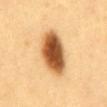Impression:
The lesion was photographed on a routine skin check and not biopsied; there is no pathology result.
Clinical summary:
The lesion is on the front of the torso. Captured under cross-polarized illumination. A region of skin cropped from a whole-body photographic capture, roughly 15 mm wide. Approximately 6 mm at its widest. A male subject, in their 60s. The total-body-photography lesion software estimated a lesion color around L≈55 a*≈23 b*≈42 in CIELAB, a lesion–skin lightness drop of about 23, and a normalized lesion–skin contrast near 13.5. The software also gave a border-irregularity rating of about 1.5/10, internal color variation of about 7.5 on a 0–10 scale, and peripheral color asymmetry of about 2. And it measured an automated nevus-likeness rating near 100 out of 100 and a detector confidence of about 100 out of 100 that the crop contains a lesion.